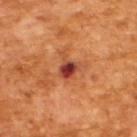biopsy status: total-body-photography surveillance lesion; no biopsy | lesion diameter: ~3.5 mm (longest diameter) | automated metrics: a lesion area of about 5.5 mm², an outline eccentricity of about 0.7 (0 = round, 1 = elongated), and a shape-asymmetry score of about 0.45 (0 = symmetric); a border-irregularity rating of about 5/10, a within-lesion color-variation index near 7/10, and radial color variation of about 2 | illumination: cross-polarized illumination | imaging modality: ~15 mm tile from a whole-body skin photo | subject: male, about 65 years old.